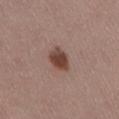The lesion was photographed on a routine skin check and not biopsied; there is no pathology result.
The total-body-photography lesion software estimated a mean CIELAB color near L≈43 a*≈20 b*≈24, about 13 CIELAB-L* units darker than the surrounding skin, and a normalized border contrast of about 10. And it measured a border-irregularity index near 2.5/10, internal color variation of about 4 on a 0–10 scale, and radial color variation of about 1. And it measured a detector confidence of about 100 out of 100 that the crop contains a lesion.
A 15 mm crop from a total-body photograph taken for skin-cancer surveillance.
About 3 mm across.
Captured under white-light illumination.
From the back.
A female subject, aged 53–57.